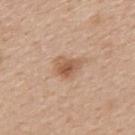<record>
<biopsy_status>not biopsied; imaged during a skin examination</biopsy_status>
<image>
  <source>total-body photography crop</source>
  <field_of_view_mm>15</field_of_view_mm>
</image>
<lesion_size>
  <long_diameter_mm_approx>3.5</long_diameter_mm_approx>
</lesion_size>
<automated_metrics>
  <cielab_L>57</cielab_L>
  <cielab_a>20</cielab_a>
  <cielab_b>32</cielab_b>
  <vs_skin_darker_L>10.0</vs_skin_darker_L>
</automated_metrics>
<patient>
  <sex>male</sex>
  <age_approx>50</age_approx>
</patient>
<lighting>white-light</lighting>
<site>mid back</site>
</record>